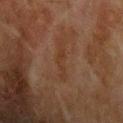Q: Was this lesion biopsied?
A: imaged on a skin check; not biopsied
Q: Lesion size?
A: ≈4.5 mm
Q: What are the patient's age and sex?
A: male, roughly 70 years of age
Q: What lighting was used for the tile?
A: cross-polarized illumination
Q: How was this image acquired?
A: 15 mm crop, total-body photography
Q: What is the anatomic site?
A: the upper back